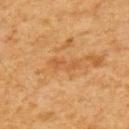workup — imaged on a skin check; not biopsied
subject — male, in their 60s
imaging modality — 15 mm crop, total-body photography
size — about 3.5 mm
body site — the upper back